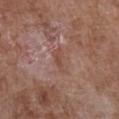follow-up: total-body-photography surveillance lesion; no biopsy
subject: female, aged 78–82
body site: the chest
automated metrics: a lesion color around L≈48 a*≈20 b*≈26 in CIELAB and a normalized border contrast of about 5.5; a border-irregularity index near 4/10, a within-lesion color-variation index near 1/10, and peripheral color asymmetry of about 0.5
image source: 15 mm crop, total-body photography
lighting: white-light illumination